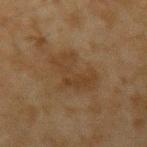* image — 15 mm crop, total-body photography
* location — the arm
* patient — male, aged around 45